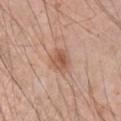A male subject aged around 45. Located on the left upper arm. A roughly 15 mm field-of-view crop from a total-body skin photograph. Approximately 3 mm at its widest. Captured under white-light illumination.